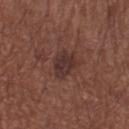Impression:
Part of a total-body skin-imaging series; this lesion was reviewed on a skin check and was not flagged for biopsy.
Context:
A female patient about 55 years old. Cropped from a total-body skin-imaging series; the visible field is about 15 mm. The lesion is on the left thigh.